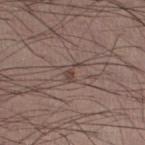Part of a total-body skin-imaging series; this lesion was reviewed on a skin check and was not flagged for biopsy.
Automated image analysis of the tile measured a footprint of about 3 mm², an eccentricity of roughly 0.85, and a symmetry-axis asymmetry near 0.5. The analysis additionally found a mean CIELAB color near L≈43 a*≈15 b*≈20 and a lesion-to-skin contrast of about 6 (normalized; higher = more distinct). It also reported a border-irregularity index near 6/10 and peripheral color asymmetry of about 0.
A lesion tile, about 15 mm wide, cut from a 3D total-body photograph.
The tile uses white-light illumination.
From the leg.
Longest diameter approximately 3 mm.
A male patient about 30 years old.